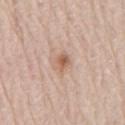| feature | finding |
|---|---|
| notes | total-body-photography surveillance lesion; no biopsy |
| image | ~15 mm tile from a whole-body skin photo |
| body site | the lower back |
| illumination | white-light |
| subject | male, aged around 80 |
| lesion diameter | ~3 mm (longest diameter) |